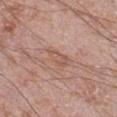The lesion was photographed on a routine skin check and not biopsied; there is no pathology result.
A 15 mm crop from a total-body photograph taken for skin-cancer surveillance.
The recorded lesion diameter is about 3.5 mm.
A male patient in their 60s.
Located on the right lower leg.
Imaged with white-light lighting.
Automated tile analysis of the lesion measured a border-irregularity index near 3.5/10, internal color variation of about 3.5 on a 0–10 scale, and peripheral color asymmetry of about 1. And it measured a classifier nevus-likeness of about 0/100 and lesion-presence confidence of about 100/100.